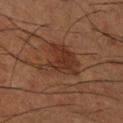Impression:
No biopsy was performed on this lesion — it was imaged during a full skin examination and was not determined to be concerning.
Background:
A male subject, aged 63–67. The lesion-visualizer software estimated a lesion area of about 12 mm², an outline eccentricity of about 0.45 (0 = round, 1 = elongated), and a shape-asymmetry score of about 0.45 (0 = symmetric). The lesion is located on the right lower leg. Cropped from a whole-body photographic skin survey; the tile spans about 15 mm. Approximately 5 mm at its widest. The tile uses cross-polarized illumination.